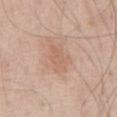Q: Was this lesion biopsied?
A: total-body-photography surveillance lesion; no biopsy
Q: How was the tile lit?
A: white-light
Q: What are the patient's age and sex?
A: male, aged 68 to 72
Q: What is the imaging modality?
A: 15 mm crop, total-body photography
Q: How large is the lesion?
A: ~4 mm (longest diameter)
Q: Where on the body is the lesion?
A: the front of the torso